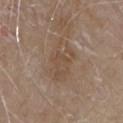Impression:
No biopsy was performed on this lesion — it was imaged during a full skin examination and was not determined to be concerning.
Context:
The lesion is located on the chest. This is a white-light tile. A 15 mm crop from a total-body photograph taken for skin-cancer surveillance. Longest diameter approximately 5 mm. The subject is a male aged around 80.